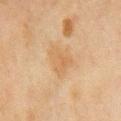Background: An algorithmic analysis of the crop reported an area of roughly 8.5 mm² and a shape-asymmetry score of about 0.4 (0 = symmetric). And it measured a lesion color around L≈52 a*≈15 b*≈33 in CIELAB, roughly 5 lightness units darker than nearby skin, and a lesion-to-skin contrast of about 5 (normalized; higher = more distinct). From the chest. A female patient, approximately 50 years of age. The tile uses cross-polarized illumination. Approximately 3.5 mm at its widest. A lesion tile, about 15 mm wide, cut from a 3D total-body photograph.